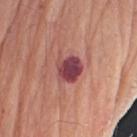Imaged during a routine full-body skin examination; the lesion was not biopsied and no histopathology is available.
Imaged with white-light lighting.
The lesion is on the right upper arm.
A 15 mm close-up extracted from a 3D total-body photography capture.
The recorded lesion diameter is about 3.5 mm.
A male subject, aged around 80.